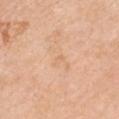diameter: ~1.5 mm (longest diameter); acquisition: ~15 mm tile from a whole-body skin photo; patient: female, aged around 55; body site: the front of the torso; tile lighting: white-light.